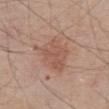{"biopsy_status": "not biopsied; imaged during a skin examination", "lighting": "white-light", "site": "abdomen", "automated_metrics": {"color_variation_0_10": 2.5, "peripheral_color_asymmetry": 1.0, "nevus_likeness_0_100": 20, "lesion_detection_confidence_0_100": 100}, "lesion_size": {"long_diameter_mm_approx": 6.0}, "image": {"source": "total-body photography crop", "field_of_view_mm": 15}, "patient": {"sex": "male", "age_approx": 80}}